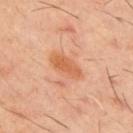Findings:
• follow-up · total-body-photography surveillance lesion; no biopsy
• automated metrics · a lesion area of about 8 mm² and an outline eccentricity of about 0.85 (0 = round, 1 = elongated); a border-irregularity rating of about 2/10, a within-lesion color-variation index near 3/10, and radial color variation of about 1; a classifier nevus-likeness of about 45/100 and a lesion-detection confidence of about 100/100
• anatomic site · the upper back
• imaging modality · 15 mm crop, total-body photography
• patient · male, aged approximately 60